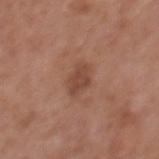biopsy_status: not biopsied; imaged during a skin examination
lighting: white-light
patient:
  sex: male
  age_approx: 70
site: mid back
lesion_size:
  long_diameter_mm_approx: 3.0
automated_metrics:
  cielab_L: 46
  cielab_a: 22
  cielab_b: 28
  nevus_likeness_0_100: 20
image:
  source: total-body photography crop
  field_of_view_mm: 15